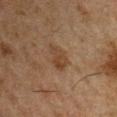Case summary:
– biopsy status · total-body-photography surveillance lesion; no biopsy
– patient · male, roughly 50 years of age
– site · the left upper arm
– lighting · cross-polarized illumination
– image · ~15 mm tile from a whole-body skin photo
– size · ~3.5 mm (longest diameter)
– automated lesion analysis · an area of roughly 5 mm², an eccentricity of roughly 0.8, and a shape-asymmetry score of about 0.35 (0 = symmetric); a border-irregularity index near 3.5/10 and internal color variation of about 2.5 on a 0–10 scale